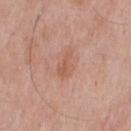• workup · catalogued during a skin exam; not biopsied
• body site · the chest
• image · 15 mm crop, total-body photography
• subject · male, aged 53–57